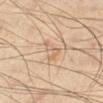This lesion was catalogued during total-body skin photography and was not selected for biopsy.
From the leg.
The recorded lesion diameter is about 3.5 mm.
A male patient aged approximately 55.
Cropped from a total-body skin-imaging series; the visible field is about 15 mm.
The lesion-visualizer software estimated a shape eccentricity near 0.85 and a shape-asymmetry score of about 0.5 (0 = symmetric). The analysis additionally found a mean CIELAB color near L≈66 a*≈16 b*≈34 and about 7 CIELAB-L* units darker than the surrounding skin. And it measured border irregularity of about 5 on a 0–10 scale and internal color variation of about 2.5 on a 0–10 scale. The software also gave an automated nevus-likeness rating near 0 out of 100 and a lesion-detection confidence of about 95/100.